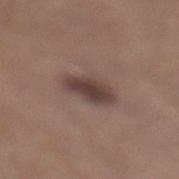Assessment:
The lesion was tiled from a total-body skin photograph and was not biopsied.
Background:
On the left lower leg. Cropped from a whole-body photographic skin survey; the tile spans about 15 mm. The recorded lesion diameter is about 4.5 mm. Captured under white-light illumination. The subject is a male roughly 30 years of age. Automated tile analysis of the lesion measured an area of roughly 8.5 mm², a shape eccentricity near 0.85, and a shape-asymmetry score of about 0.2 (0 = symmetric). And it measured a border-irregularity index near 2.5/10, a within-lesion color-variation index near 3/10, and peripheral color asymmetry of about 1.